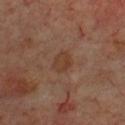follow-up: imaged on a skin check; not biopsied
TBP lesion metrics: a lesion color around L≈36 a*≈18 b*≈27 in CIELAB and a normalized lesion–skin contrast near 6; a color-variation rating of about 2/10 and radial color variation of about 0.5; a classifier nevus-likeness of about 5/100
anatomic site: the chest
patient: male, roughly 70 years of age
image: ~15 mm crop, total-body skin-cancer survey
lesion diameter: about 2.5 mm